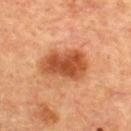notes: total-body-photography surveillance lesion; no biopsy | lesion size: about 5.5 mm | illumination: cross-polarized | body site: the upper back | acquisition: total-body-photography crop, ~15 mm field of view | patient: female, approximately 55 years of age.